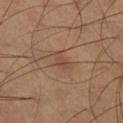This lesion was catalogued during total-body skin photography and was not selected for biopsy. From the leg. Cropped from a whole-body photographic skin survey; the tile spans about 15 mm. The patient is a male about 60 years old. Imaged with cross-polarized lighting. Approximately 3 mm at its widest.